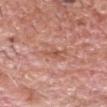Q: Was this lesion biopsied?
A: catalogued during a skin exam; not biopsied
Q: Lesion location?
A: the head or neck
Q: What is the imaging modality?
A: 15 mm crop, total-body photography
Q: Patient demographics?
A: male, aged approximately 60